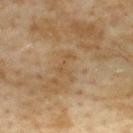Recorded during total-body skin imaging; not selected for excision or biopsy. On the upper back. A lesion tile, about 15 mm wide, cut from a 3D total-body photograph. The patient is a female in their 60s. Captured under cross-polarized illumination. The recorded lesion diameter is about 3.5 mm.